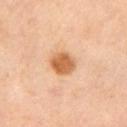Case summary:
• workup: total-body-photography surveillance lesion; no biopsy
• diameter: ≈3 mm
• subject: female, aged approximately 45
• site: the left upper arm
• tile lighting: cross-polarized illumination
• image source: 15 mm crop, total-body photography
• TBP lesion metrics: internal color variation of about 3.5 on a 0–10 scale and a peripheral color-asymmetry measure near 1; a classifier nevus-likeness of about 100/100 and a lesion-detection confidence of about 100/100